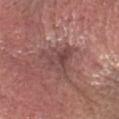Notes:
• notes · imaged on a skin check; not biopsied
• lesion size · about 4.5 mm
• body site · the head or neck
• tile lighting · white-light illumination
• patient · male, about 75 years old
• automated metrics · an area of roughly 10 mm², an eccentricity of roughly 0.45, and a symmetry-axis asymmetry near 0.55; border irregularity of about 6 on a 0–10 scale and a color-variation rating of about 5.5/10; an automated nevus-likeness rating near 0 out of 100 and a lesion-detection confidence of about 75/100
• image source · total-body-photography crop, ~15 mm field of view